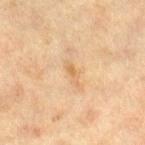Captured during whole-body skin photography for melanoma surveillance; the lesion was not biopsied. Measured at roughly 3 mm in maximum diameter. Automated tile analysis of the lesion measured an eccentricity of roughly 0.9. It also reported a lesion color around L≈58 a*≈16 b*≈35 in CIELAB and roughly 7 lightness units darker than nearby skin. A 15 mm close-up extracted from a 3D total-body photography capture. The tile uses cross-polarized illumination. A female patient, approximately 40 years of age. From the right lower leg.